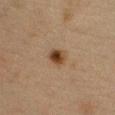Part of a total-body skin-imaging series; this lesion was reviewed on a skin check and was not flagged for biopsy.
From the chest.
A female subject, in their 40s.
This image is a 15 mm lesion crop taken from a total-body photograph.